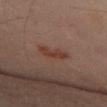follow-up=imaged on a skin check; not biopsied.